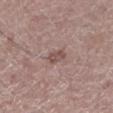The lesion was photographed on a routine skin check and not biopsied; there is no pathology result. The total-body-photography lesion software estimated an area of roughly 3.5 mm², a shape eccentricity near 0.85, and a shape-asymmetry score of about 0.3 (0 = symmetric). It also reported an average lesion color of about L≈50 a*≈18 b*≈21 (CIELAB), roughly 9 lightness units darker than nearby skin, and a normalized lesion–skin contrast near 6.5. The software also gave a border-irregularity index near 2.5/10 and a color-variation rating of about 2/10. It also reported an automated nevus-likeness rating near 0 out of 100 and a detector confidence of about 100 out of 100 that the crop contains a lesion. A close-up tile cropped from a whole-body skin photograph, about 15 mm across. The tile uses white-light illumination. Approximately 2.5 mm at its widest. The lesion is located on the left lower leg. The patient is a male aged around 65.